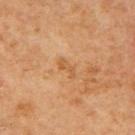Part of a total-body skin-imaging series; this lesion was reviewed on a skin check and was not flagged for biopsy. On the mid back. The subject is a male approximately 65 years of age. Cropped from a total-body skin-imaging series; the visible field is about 15 mm.